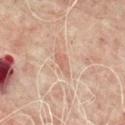Impression: Part of a total-body skin-imaging series; this lesion was reviewed on a skin check and was not flagged for biopsy. Image and clinical context: A male patient approximately 70 years of age. A lesion tile, about 15 mm wide, cut from a 3D total-body photograph. Located on the mid back. Automated image analysis of the tile measured border irregularity of about 4 on a 0–10 scale, a color-variation rating of about 0.5/10, and peripheral color asymmetry of about 0.